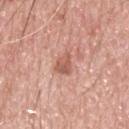No biopsy was performed on this lesion — it was imaged during a full skin examination and was not determined to be concerning.
Located on the upper back.
A close-up tile cropped from a whole-body skin photograph, about 15 mm across.
The subject is a male about 70 years old.
Automated tile analysis of the lesion measured a lesion area of about 4 mm², an outline eccentricity of about 0.8 (0 = round, 1 = elongated), and a shape-asymmetry score of about 0.35 (0 = symmetric). And it measured an average lesion color of about L≈57 a*≈25 b*≈29 (CIELAB) and about 11 CIELAB-L* units darker than the surrounding skin. The analysis additionally found internal color variation of about 3 on a 0–10 scale. The analysis additionally found an automated nevus-likeness rating near 10 out of 100.
This is a white-light tile.